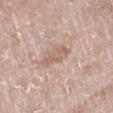{
  "biopsy_status": "not biopsied; imaged during a skin examination",
  "site": "leg",
  "image": {
    "source": "total-body photography crop",
    "field_of_view_mm": 15
  },
  "patient": {
    "sex": "female",
    "age_approx": 75
  },
  "lighting": "white-light"
}